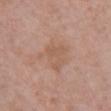| field | value |
|---|---|
| workup | imaged on a skin check; not biopsied |
| location | the front of the torso |
| patient | female, roughly 50 years of age |
| acquisition | total-body-photography crop, ~15 mm field of view |
| lesion diameter | ≈4.5 mm |
| tile lighting | white-light |